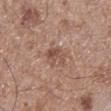  biopsy_status: not biopsied; imaged during a skin examination
  patient:
    sex: male
    age_approx: 60
  image:
    source: total-body photography crop
    field_of_view_mm: 15
  site: right lower leg